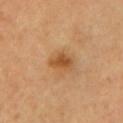biopsy_status: not biopsied; imaged during a skin examination
image:
  source: total-body photography crop
  field_of_view_mm: 15
patient:
  sex: female
site: left upper arm
automated_metrics:
  cielab_L: 51
  cielab_a: 23
  cielab_b: 41
  vs_skin_darker_L: 11.0
  vs_skin_contrast_norm: 8.0
  nevus_likeness_0_100: 55
  lesion_detection_confidence_0_100: 100
lesion_size:
  long_diameter_mm_approx: 3.0
lighting: cross-polarized